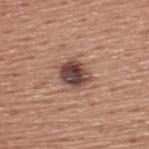biopsy status: catalogued during a skin exam; not biopsied
acquisition: total-body-photography crop, ~15 mm field of view
body site: the upper back
subject: male, aged 43–47
lesion size: about 3.5 mm
illumination: white-light
image-analysis metrics: a mean CIELAB color near L≈44 a*≈19 b*≈23, a lesion–skin lightness drop of about 16, and a lesion-to-skin contrast of about 12 (normalized; higher = more distinct); a border-irregularity rating of about 2/10; a classifier nevus-likeness of about 25/100 and a lesion-detection confidence of about 100/100